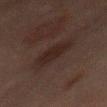Cropped from a whole-body photographic skin survey; the tile spans about 15 mm. Captured under cross-polarized illumination. The lesion's longest dimension is about 6 mm. A male patient roughly 65 years of age. The lesion is on the front of the torso.